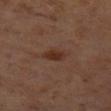<lesion>
  <biopsy_status>not biopsied; imaged during a skin examination</biopsy_status>
  <lesion_size>
    <long_diameter_mm_approx>3.0</long_diameter_mm_approx>
  </lesion_size>
  <site>left thigh</site>
  <patient>
    <sex>male</sex>
    <age_approx>60</age_approx>
  </patient>
  <lighting>cross-polarized</lighting>
  <image>
    <source>total-body photography crop</source>
    <field_of_view_mm>15</field_of_view_mm>
  </image>
  <automated_metrics>
    <area_mm2_approx>4.0</area_mm2_approx>
    <shape_asymmetry>0.2</shape_asymmetry>
    <border_irregularity_0_10>2.0</border_irregularity_0_10>
    <color_variation_0_10>2.0</color_variation_0_10>
    <peripheral_color_asymmetry>1.0</peripheral_color_asymmetry>
  </automated_metrics>
</lesion>